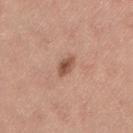{
  "biopsy_status": "not biopsied; imaged during a skin examination",
  "image": {
    "source": "total-body photography crop",
    "field_of_view_mm": 15
  },
  "patient": {
    "sex": "female",
    "age_approx": 25
  },
  "site": "left thigh",
  "automated_metrics": {
    "cielab_L": 53,
    "cielab_a": 22,
    "cielab_b": 29,
    "vs_skin_darker_L": 12.0,
    "vs_skin_contrast_norm": 8.0,
    "border_irregularity_0_10": 2.5,
    "color_variation_0_10": 2.5,
    "peripheral_color_asymmetry": 1.0
  },
  "lighting": "white-light"
}